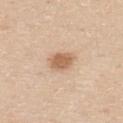Impression: Part of a total-body skin-imaging series; this lesion was reviewed on a skin check and was not flagged for biopsy. Background: A lesion tile, about 15 mm wide, cut from a 3D total-body photograph. Located on the upper back. This is a white-light tile. Approximately 3 mm at its widest. A male subject, approximately 30 years of age.